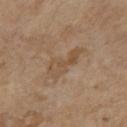The lesion was tiled from a total-body skin photograph and was not biopsied. The tile uses white-light illumination. Cropped from a total-body skin-imaging series; the visible field is about 15 mm. The lesion is located on the chest. About 5.5 mm across. A female subject aged 63–67. An algorithmic analysis of the crop reported an average lesion color of about L≈51 a*≈15 b*≈31 (CIELAB), about 6 CIELAB-L* units darker than the surrounding skin, and a lesion-to-skin contrast of about 5 (normalized; higher = more distinct). The software also gave an automated nevus-likeness rating near 0 out of 100 and lesion-presence confidence of about 100/100.